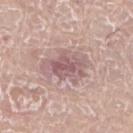Imaged during a routine full-body skin examination; the lesion was not biopsied and no histopathology is available.
The subject is a male aged around 75.
This is a white-light tile.
Measured at roughly 5 mm in maximum diameter.
On the leg.
A region of skin cropped from a whole-body photographic capture, roughly 15 mm wide.